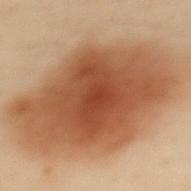Recorded during total-body skin imaging; not selected for excision or biopsy.
About 5.5 mm across.
Automated image analysis of the tile measured an outline eccentricity of about 0.6 (0 = round, 1 = elongated) and two-axis asymmetry of about 0.25. And it measured a border-irregularity rating of about 3.5/10 and a color-variation rating of about 3.5/10.
This is a cross-polarized tile.
Located on the upper back.
A lesion tile, about 15 mm wide, cut from a 3D total-body photograph.
A female subject about 60 years old.